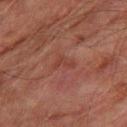{
  "biopsy_status": "not biopsied; imaged during a skin examination",
  "image": {
    "source": "total-body photography crop",
    "field_of_view_mm": 15
  },
  "patient": {
    "sex": "male",
    "age_approx": 75
  },
  "site": "right thigh",
  "lesion_size": {
    "long_diameter_mm_approx": 2.5
  },
  "lighting": "cross-polarized",
  "automated_metrics": {
    "eccentricity": 0.8,
    "vs_skin_darker_L": 5.0
  }
}